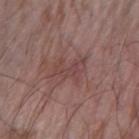Assessment:
The lesion was photographed on a routine skin check and not biopsied; there is no pathology result.
Clinical summary:
The patient is a male aged around 65. Cropped from a total-body skin-imaging series; the visible field is about 15 mm. Approximately 4 mm at its widest. This is a white-light tile. The total-body-photography lesion software estimated an eccentricity of roughly 0.8 and a shape-asymmetry score of about 0.35 (0 = symmetric). The software also gave a nevus-likeness score of about 0/100. Located on the right forearm.